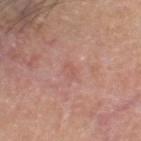| key | value |
|---|---|
| follow-up | imaged on a skin check; not biopsied |
| TBP lesion metrics | a border-irregularity index near 2.5/10 and radial color variation of about 0; an automated nevus-likeness rating near 0 out of 100 |
| imaging modality | total-body-photography crop, ~15 mm field of view |
| tile lighting | white-light |
| site | the head or neck |
| size | ~2 mm (longest diameter) |
| subject | male, in their mid-50s |